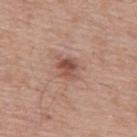biopsy status: no biopsy performed (imaged during a skin exam) | acquisition: total-body-photography crop, ~15 mm field of view | patient: male, roughly 55 years of age | body site: the mid back | lighting: white-light.